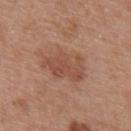No biopsy was performed on this lesion — it was imaged during a full skin examination and was not determined to be concerning.
The lesion's longest dimension is about 5 mm.
Cropped from a total-body skin-imaging series; the visible field is about 15 mm.
The lesion-visualizer software estimated a lesion area of about 13 mm², an outline eccentricity of about 0.75 (0 = round, 1 = elongated), and two-axis asymmetry of about 0.3. It also reported border irregularity of about 3.5 on a 0–10 scale, a color-variation rating of about 3/10, and a peripheral color-asymmetry measure near 1. It also reported a nevus-likeness score of about 15/100 and a lesion-detection confidence of about 100/100.
The lesion is on the upper back.
A male subject aged around 30.